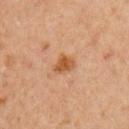This lesion was catalogued during total-body skin photography and was not selected for biopsy. A male subject, roughly 60 years of age. The lesion's longest dimension is about 3 mm. A 15 mm close-up tile from a total-body photography series done for melanoma screening. The lesion is located on the left upper arm. Imaged with cross-polarized lighting.